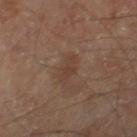Part of a total-body skin-imaging series; this lesion was reviewed on a skin check and was not flagged for biopsy. A male patient, aged 68–72. A 15 mm close-up extracted from a 3D total-body photography capture. From the right thigh. The recorded lesion diameter is about 3 mm.